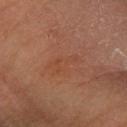Recorded during total-body skin imaging; not selected for excision or biopsy.
Cropped from a total-body skin-imaging series; the visible field is about 15 mm.
The lesion is located on the left forearm.
Automated image analysis of the tile measured a lesion area of about 6 mm², an eccentricity of roughly 0.9, and a shape-asymmetry score of about 0.4 (0 = symmetric).
Captured under cross-polarized illumination.
Approximately 4 mm at its widest.
A male patient, aged 58–62.